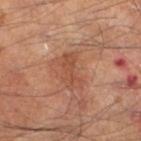{"biopsy_status": "not biopsied; imaged during a skin examination", "lesion_size": {"long_diameter_mm_approx": 4.0}, "patient": {"sex": "male", "age_approx": 65}, "automated_metrics": {"area_mm2_approx": 6.0, "eccentricity": 0.9, "shape_asymmetry": 0.35, "nevus_likeness_0_100": 0}, "image": {"source": "total-body photography crop", "field_of_view_mm": 15}, "lighting": "cross-polarized", "site": "left lower leg"}